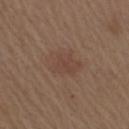Q: Was this lesion biopsied?
A: total-body-photography surveillance lesion; no biopsy
Q: What are the patient's age and sex?
A: male, approximately 70 years of age
Q: Automated lesion metrics?
A: a footprint of about 8 mm², an outline eccentricity of about 0.65 (0 = round, 1 = elongated), and a symmetry-axis asymmetry near 0.3; border irregularity of about 3 on a 0–10 scale and radial color variation of about 0.5
Q: How large is the lesion?
A: about 3.5 mm
Q: Where on the body is the lesion?
A: the arm
Q: How was the tile lit?
A: white-light illumination
Q: How was this image acquired?
A: ~15 mm tile from a whole-body skin photo